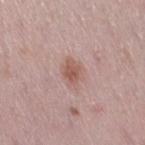Q: Is there a histopathology result?
A: no biopsy performed (imaged during a skin exam)
Q: Lesion location?
A: the right thigh
Q: What are the patient's age and sex?
A: female, aged approximately 30
Q: What is the imaging modality?
A: ~15 mm crop, total-body skin-cancer survey